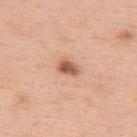The lesion was tiled from a total-body skin photograph and was not biopsied.
From the upper back.
An algorithmic analysis of the crop reported an area of roughly 4 mm², an outline eccentricity of about 0.75 (0 = round, 1 = elongated), and a symmetry-axis asymmetry near 0.25. The analysis additionally found a mean CIELAB color near L≈58 a*≈24 b*≈34, roughly 14 lightness units darker than nearby skin, and a normalized lesion–skin contrast near 9.
A close-up tile cropped from a whole-body skin photograph, about 15 mm across.
The patient is a male about 60 years old.
The tile uses white-light illumination.
The recorded lesion diameter is about 2.5 mm.